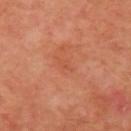  biopsy_status: not biopsied; imaged during a skin examination
  patient:
    sex: male
    age_approx: 50
  site: left upper arm
  image:
    source: total-body photography crop
    field_of_view_mm: 15
  lighting: cross-polarized